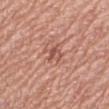Q: Was this lesion biopsied?
A: imaged on a skin check; not biopsied
Q: How large is the lesion?
A: ~2.5 mm (longest diameter)
Q: Patient demographics?
A: female, aged around 65
Q: Lesion location?
A: the arm
Q: What kind of image is this?
A: 15 mm crop, total-body photography
Q: How was the tile lit?
A: white-light
Q: What did automated image analysis measure?
A: an area of roughly 3.5 mm², an outline eccentricity of about 0.8 (0 = round, 1 = elongated), and two-axis asymmetry of about 0.4